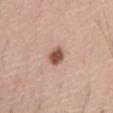On the abdomen.
Cropped from a whole-body photographic skin survey; the tile spans about 15 mm.
A male subject in their 50s.